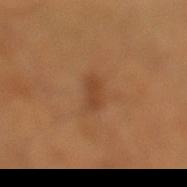| key | value |
|---|---|
| biopsy status | no biopsy performed (imaged during a skin exam) |
| image | 15 mm crop, total-body photography |
| automated metrics | internal color variation of about 1.5 on a 0–10 scale; a classifier nevus-likeness of about 45/100 and a lesion-detection confidence of about 100/100 |
| illumination | cross-polarized |
| subject | male, aged 53 to 57 |
| anatomic site | the left lower leg |